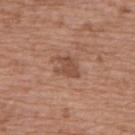This lesion was catalogued during total-body skin photography and was not selected for biopsy. Automated tile analysis of the lesion measured a footprint of about 5 mm², an outline eccentricity of about 0.7 (0 = round, 1 = elongated), and two-axis asymmetry of about 0.3. The software also gave a lesion–skin lightness drop of about 9 and a lesion-to-skin contrast of about 6.5 (normalized; higher = more distinct). It also reported internal color variation of about 1 on a 0–10 scale and a peripheral color-asymmetry measure near 0.5. Captured under white-light illumination. The lesion is on the upper back. Cropped from a total-body skin-imaging series; the visible field is about 15 mm. A female patient about 75 years old. Longest diameter approximately 3 mm.